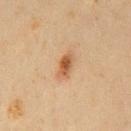Q: Was this lesion biopsied?
A: no biopsy performed (imaged during a skin exam)
Q: Who is the patient?
A: male, approximately 60 years of age
Q: Lesion location?
A: the chest
Q: How was this image acquired?
A: 15 mm crop, total-body photography
Q: Automated lesion metrics?
A: a footprint of about 4 mm²; an average lesion color of about L≈46 a*≈20 b*≈32 (CIELAB), a lesion–skin lightness drop of about 11, and a lesion-to-skin contrast of about 8.5 (normalized; higher = more distinct); border irregularity of about 3 on a 0–10 scale, a color-variation rating of about 3/10, and radial color variation of about 0.5
Q: How was the tile lit?
A: cross-polarized